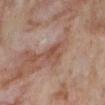workup=catalogued during a skin exam; not biopsied
subject=female, aged 53 to 57
anatomic site=the leg
lighting=cross-polarized illumination
imaging modality=~15 mm crop, total-body skin-cancer survey